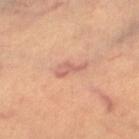Q: Illumination type?
A: cross-polarized illumination
Q: What kind of image is this?
A: ~15 mm tile from a whole-body skin photo
Q: Automated lesion metrics?
A: a footprint of about 3.5 mm², an outline eccentricity of about 0.9 (0 = round, 1 = elongated), and a shape-asymmetry score of about 0.45 (0 = symmetric); radial color variation of about 0.5
Q: Where on the body is the lesion?
A: the right thigh
Q: What are the patient's age and sex?
A: female, aged around 30
Q: Lesion size?
A: ≈3.5 mm